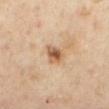Case summary:
– follow-up: imaged on a skin check; not biopsied
– size: ≈2.5 mm
– body site: the mid back
– image source: total-body-photography crop, ~15 mm field of view
– patient: female, about 60 years old
– automated lesion analysis: a footprint of about 5.5 mm², a shape eccentricity near 0.45, and a shape-asymmetry score of about 0.25 (0 = symmetric); an average lesion color of about L≈58 a*≈19 b*≈35 (CIELAB); a nevus-likeness score of about 85/100 and a lesion-detection confidence of about 100/100